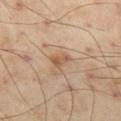{"biopsy_status": "not biopsied; imaged during a skin examination", "patient": {"sex": "male", "age_approx": 55}, "lesion_size": {"long_diameter_mm_approx": 2.5}, "image": {"source": "total-body photography crop", "field_of_view_mm": 15}, "lighting": "cross-polarized", "site": "left thigh"}